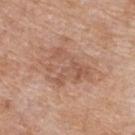Part of a total-body skin-imaging series; this lesion was reviewed on a skin check and was not flagged for biopsy. From the upper back. The recorded lesion diameter is about 5.5 mm. Automated tile analysis of the lesion measured an area of roughly 14 mm² and an eccentricity of roughly 0.7. The analysis additionally found an average lesion color of about L≈56 a*≈21 b*≈29 (CIELAB), roughly 8 lightness units darker than nearby skin, and a normalized lesion–skin contrast near 5.5. And it measured a border-irregularity rating of about 7/10, a color-variation rating of about 4.5/10, and a peripheral color-asymmetry measure near 1.5. The software also gave a classifier nevus-likeness of about 0/100 and a lesion-detection confidence of about 100/100. Imaged with white-light lighting. A 15 mm close-up tile from a total-body photography series done for melanoma screening. The patient is a female in their mid-70s.